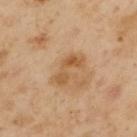The lesion was photographed on a routine skin check and not biopsied; there is no pathology result. A 15 mm crop from a total-body photograph taken for skin-cancer surveillance. The total-body-photography lesion software estimated border irregularity of about 6.5 on a 0–10 scale and peripheral color asymmetry of about 1. The analysis additionally found a nevus-likeness score of about 0/100 and lesion-presence confidence of about 100/100. The lesion's longest dimension is about 4 mm. A male patient, in their mid- to late 50s. Captured under cross-polarized illumination. The lesion is on the left upper arm.